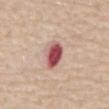Impression: The lesion was photographed on a routine skin check and not biopsied; there is no pathology result. Acquisition and patient details: The lesion's longest dimension is about 3.5 mm. Cropped from a whole-body photographic skin survey; the tile spans about 15 mm. This is a white-light tile. A male subject aged approximately 75. Located on the mid back.